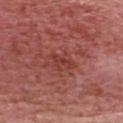Impression:
Recorded during total-body skin imaging; not selected for excision or biopsy.
Clinical summary:
Measured at roughly 3 mm in maximum diameter. A male subject in their 60s. This is a white-light tile. This image is a 15 mm lesion crop taken from a total-body photograph. An algorithmic analysis of the crop reported an average lesion color of about L≈40 a*≈31 b*≈27 (CIELAB), a lesion–skin lightness drop of about 7, and a normalized lesion–skin contrast near 6. The software also gave a border-irregularity index near 5/10 and a within-lesion color-variation index near 0.5/10. It also reported lesion-presence confidence of about 100/100. The lesion is on the head or neck.